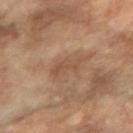No biopsy was performed on this lesion — it was imaged during a full skin examination and was not determined to be concerning. This image is a 15 mm lesion crop taken from a total-body photograph. Located on the right forearm. A female subject, aged approximately 70.